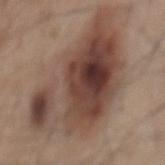workup: total-body-photography surveillance lesion; no biopsy | site: the mid back | subject: male, aged 53 to 57 | lesion size: ~12 mm (longest diameter) | tile lighting: white-light | automated lesion analysis: a footprint of about 65 mm², an eccentricity of roughly 0.75, and a symmetry-axis asymmetry near 0.35; an average lesion color of about L≈44 a*≈17 b*≈24 (CIELAB), roughly 13 lightness units darker than nearby skin, and a lesion-to-skin contrast of about 10 (normalized; higher = more distinct); border irregularity of about 4.5 on a 0–10 scale, internal color variation of about 10 on a 0–10 scale, and a peripheral color-asymmetry measure near 4; a classifier nevus-likeness of about 80/100 and a lesion-detection confidence of about 100/100 | acquisition: 15 mm crop, total-body photography.